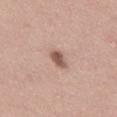biopsy status: total-body-photography surveillance lesion; no biopsy | anatomic site: the back | imaging modality: ~15 mm tile from a whole-body skin photo | subject: female, in their mid-20s.